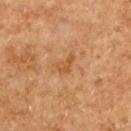<case>
  <biopsy_status>not biopsied; imaged during a skin examination</biopsy_status>
  <site>upper back</site>
  <automated_metrics>
    <area_mm2_approx>3.5</area_mm2_approx>
    <eccentricity>0.8</eccentricity>
    <shape_asymmetry>0.45</shape_asymmetry>
  </automated_metrics>
  <image>
    <source>total-body photography crop</source>
    <field_of_view_mm>15</field_of_view_mm>
  </image>
  <lesion_size>
    <long_diameter_mm_approx>2.5</long_diameter_mm_approx>
  </lesion_size>
  <patient>
    <sex>female</sex>
    <age_approx>60</age_approx>
  </patient>
</case>